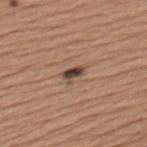The lesion was tiled from a total-body skin photograph and was not biopsied.
A 15 mm close-up tile from a total-body photography series done for melanoma screening.
A male patient in their mid-40s.
From the left upper arm.